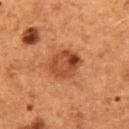This lesion was catalogued during total-body skin photography and was not selected for biopsy.
Approximately 4 mm at its widest.
The tile uses cross-polarized illumination.
On the left thigh.
A female subject aged 48–52.
This image is a 15 mm lesion crop taken from a total-body photograph.